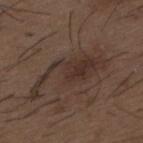A male subject about 50 years old.
Located on the mid back.
This is a white-light tile.
A roughly 15 mm field-of-view crop from a total-body skin photograph.
Automated image analysis of the tile measured a lesion area of about 15 mm², a shape eccentricity near 0.9, and two-axis asymmetry of about 0.55.